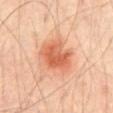Imaged during a routine full-body skin examination; the lesion was not biopsied and no histopathology is available. The lesion is located on the abdomen. A male patient, roughly 65 years of age. Imaged with cross-polarized lighting. A 15 mm close-up extracted from a 3D total-body photography capture.